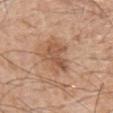Imaged during a routine full-body skin examination; the lesion was not biopsied and no histopathology is available. Imaged with white-light lighting. Automated image analysis of the tile measured a mean CIELAB color near L≈54 a*≈21 b*≈32, a lesion–skin lightness drop of about 9, and a normalized border contrast of about 6.5. It also reported border irregularity of about 4.5 on a 0–10 scale, internal color variation of about 3.5 on a 0–10 scale, and peripheral color asymmetry of about 1.5. The patient is a male aged approximately 55. A roughly 15 mm field-of-view crop from a total-body skin photograph. The lesion is on the chest.